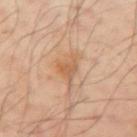A 15 mm close-up extracted from a 3D total-body photography capture. A male patient, in their 50s. The lesion is located on the left arm.